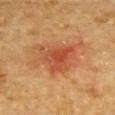Case summary:
* notes · no biopsy performed (imaged during a skin exam)
* TBP lesion metrics · an area of roughly 15 mm², an outline eccentricity of about 0.6 (0 = round, 1 = elongated), and two-axis asymmetry of about 0.4; border irregularity of about 4.5 on a 0–10 scale and peripheral color asymmetry of about 2; an automated nevus-likeness rating near 5 out of 100 and lesion-presence confidence of about 100/100
* site · the chest
* acquisition · ~15 mm crop, total-body skin-cancer survey
* patient · male, aged 83 or older
* lesion diameter · ~5 mm (longest diameter)